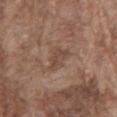No biopsy was performed on this lesion — it was imaged during a full skin examination and was not determined to be concerning.